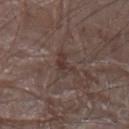Clinical impression: No biopsy was performed on this lesion — it was imaged during a full skin examination and was not determined to be concerning. Image and clinical context: This is a white-light tile. Approximately 3 mm at its widest. A region of skin cropped from a whole-body photographic capture, roughly 15 mm wide. On the right arm. The patient is a male roughly 80 years of age. The total-body-photography lesion software estimated a footprint of about 4 mm², an eccentricity of roughly 0.8, and a symmetry-axis asymmetry near 0.45. And it measured a lesion color around L≈35 a*≈15 b*≈18 in CIELAB and a normalized border contrast of about 6.